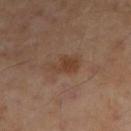biopsy status = imaged on a skin check; not biopsied
imaging modality = ~15 mm tile from a whole-body skin photo
subject = male, in their mid-50s
location = the right upper arm